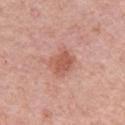A close-up tile cropped from a whole-body skin photograph, about 15 mm across. Captured under white-light illumination. About 3.5 mm across. A female patient, aged approximately 65. The total-body-photography lesion software estimated a shape eccentricity near 0.6 and a symmetry-axis asymmetry near 0.2. The analysis additionally found a lesion color around L≈57 a*≈26 b*≈30 in CIELAB, roughly 10 lightness units darker than nearby skin, and a lesion-to-skin contrast of about 7 (normalized; higher = more distinct). And it measured a classifier nevus-likeness of about 50/100 and a detector confidence of about 100 out of 100 that the crop contains a lesion. On the left upper arm.